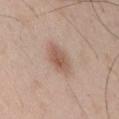{
  "biopsy_status": "not biopsied; imaged during a skin examination",
  "lesion_size": {
    "long_diameter_mm_approx": 4.0
  },
  "patient": {
    "sex": "male",
    "age_approx": 65
  },
  "lighting": "white-light",
  "image": {
    "source": "total-body photography crop",
    "field_of_view_mm": 15
  },
  "site": "abdomen"
}